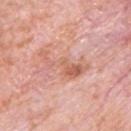workup = imaged on a skin check; not biopsied
site = the chest
image source = ~15 mm tile from a whole-body skin photo
subject = male, roughly 80 years of age
size = about 6 mm